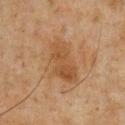  biopsy_status: not biopsied; imaged during a skin examination
  lighting: cross-polarized
  site: chest
  patient:
    sex: male
    age_approx: 60
  image:
    source: total-body photography crop
    field_of_view_mm: 15